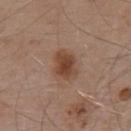Recorded during total-body skin imaging; not selected for excision or biopsy. A 15 mm crop from a total-body photograph taken for skin-cancer surveillance. The total-body-photography lesion software estimated a lesion area of about 8 mm², an outline eccentricity of about 0.65 (0 = round, 1 = elongated), and a shape-asymmetry score of about 0.2 (0 = symmetric). The analysis additionally found a nevus-likeness score of about 90/100. Approximately 3.5 mm at its widest. A male patient approximately 55 years of age. The tile uses white-light illumination. On the upper back.